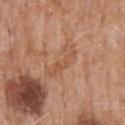notes — total-body-photography surveillance lesion; no biopsy
patient — male, about 70 years old
acquisition — ~15 mm tile from a whole-body skin photo
body site — the back
size — ~4.5 mm (longest diameter)